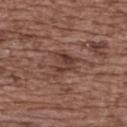Impression:
Imaged during a routine full-body skin examination; the lesion was not biopsied and no histopathology is available.
Background:
The subject is a female in their mid-70s. The lesion is located on the upper back. Approximately 3.5 mm at its widest. A 15 mm close-up extracted from a 3D total-body photography capture. This is a white-light tile. The total-body-photography lesion software estimated an area of roughly 7 mm², a shape eccentricity near 0.55, and a shape-asymmetry score of about 0.5 (0 = symmetric). And it measured a border-irregularity rating of about 5/10, a within-lesion color-variation index near 5/10, and a peripheral color-asymmetry measure near 2. The analysis additionally found a classifier nevus-likeness of about 0/100 and a lesion-detection confidence of about 65/100.